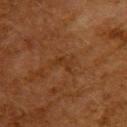{
  "biopsy_status": "not biopsied; imaged during a skin examination",
  "site": "upper back",
  "patient": {
    "sex": "female",
    "age_approx": 50
  },
  "image": {
    "source": "total-body photography crop",
    "field_of_view_mm": 15
  },
  "automated_metrics": {
    "area_mm2_approx": 3.0,
    "eccentricity": 0.85,
    "shape_asymmetry": 0.6,
    "cielab_L": 27,
    "cielab_a": 19,
    "cielab_b": 28,
    "border_irregularity_0_10": 6.0,
    "color_variation_0_10": 0.0,
    "peripheral_color_asymmetry": 0.0,
    "nevus_likeness_0_100": 0,
    "lesion_detection_confidence_0_100": 100
  },
  "lesion_size": {
    "long_diameter_mm_approx": 2.5
  },
  "lighting": "cross-polarized"
}